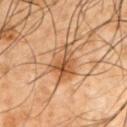Case summary:
- workup: total-body-photography surveillance lesion; no biopsy
- image: 15 mm crop, total-body photography
- tile lighting: cross-polarized illumination
- subject: male, aged approximately 50
- location: the left upper arm